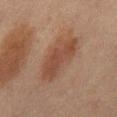Case summary:
– biopsy status — total-body-photography surveillance lesion; no biopsy
– subject — male, aged 63–67
– tile lighting — cross-polarized
– image source — ~15 mm tile from a whole-body skin photo
– lesion size — ≈6.5 mm
– body site — the front of the torso